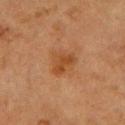The lesion was tiled from a total-body skin photograph and was not biopsied. A female patient roughly 55 years of age. A close-up tile cropped from a whole-body skin photograph, about 15 mm across. The lesion is located on the arm.